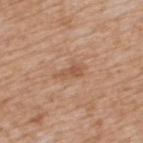notes — imaged on a skin check; not biopsied
lesion diameter — about 4 mm
lighting — white-light
patient — male, in their mid-60s
imaging modality — 15 mm crop, total-body photography
body site — the upper back